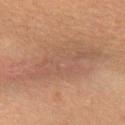Imaged during a routine full-body skin examination; the lesion was not biopsied and no histopathology is available.